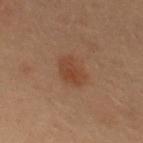Impression: This lesion was catalogued during total-body skin photography and was not selected for biopsy. Acquisition and patient details: A female subject approximately 30 years of age. Cropped from a total-body skin-imaging series; the visible field is about 15 mm. The lesion is on the upper back.